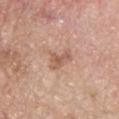This lesion was catalogued during total-body skin photography and was not selected for biopsy.
The lesion is located on the upper back.
Imaged with white-light lighting.
Approximately 3.5 mm at its widest.
This image is a 15 mm lesion crop taken from a total-body photograph.
A male subject aged approximately 65.